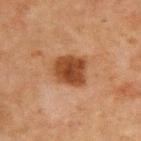| key | value |
|---|---|
| workup | no biopsy performed (imaged during a skin exam) |
| illumination | cross-polarized illumination |
| TBP lesion metrics | a footprint of about 12 mm², a shape eccentricity near 0.6, and two-axis asymmetry of about 0.2; a mean CIELAB color near L≈36 a*≈20 b*≈31, about 12 CIELAB-L* units darker than the surrounding skin, and a lesion-to-skin contrast of about 10.5 (normalized; higher = more distinct); border irregularity of about 2 on a 0–10 scale, a color-variation rating of about 3.5/10, and radial color variation of about 1; a nevus-likeness score of about 90/100 and a lesion-detection confidence of about 100/100 |
| anatomic site | the chest |
| patient | male, aged 63 to 67 |
| imaging modality | 15 mm crop, total-body photography |
| diameter | ~4 mm (longest diameter) |